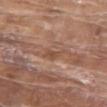body site — the front of the torso
image — 15 mm crop, total-body photography
patient — male, approximately 80 years of age
lighting — white-light illumination
lesion diameter — ~3.5 mm (longest diameter)